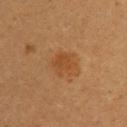Captured during whole-body skin photography for melanoma surveillance; the lesion was not biopsied.
This is a cross-polarized tile.
The lesion-visualizer software estimated border irregularity of about 3.5 on a 0–10 scale, internal color variation of about 1.5 on a 0–10 scale, and a peripheral color-asymmetry measure near 0.5. And it measured an automated nevus-likeness rating near 70 out of 100 and a detector confidence of about 100 out of 100 that the crop contains a lesion.
Cropped from a whole-body photographic skin survey; the tile spans about 15 mm.
A female subject about 20 years old.
The lesion is on the upper back.